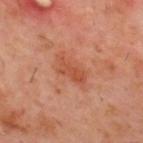* follow-up: no biopsy performed (imaged during a skin exam)
* anatomic site: the mid back
* imaging modality: 15 mm crop, total-body photography
* subject: male, roughly 60 years of age